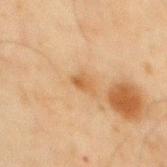Case summary:
– follow-up — imaged on a skin check; not biopsied
– patient — male, about 45 years old
– body site — the mid back
– image source — total-body-photography crop, ~15 mm field of view
– size — ~2.5 mm (longest diameter)